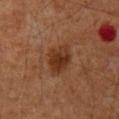Part of a total-body skin-imaging series; this lesion was reviewed on a skin check and was not flagged for biopsy.
A roughly 15 mm field-of-view crop from a total-body skin photograph.
A male patient, in their 60s.
Located on the mid back.